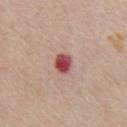Recorded during total-body skin imaging; not selected for excision or biopsy. The lesion's longest dimension is about 2.5 mm. A region of skin cropped from a whole-body photographic capture, roughly 15 mm wide. Located on the chest. The subject is a male about 65 years old. This is a white-light tile.